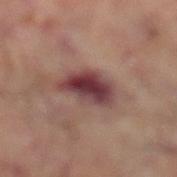- follow-up: catalogued during a skin exam; not biopsied
- image source: 15 mm crop, total-body photography
- location: the leg
- subject: male, aged 68 to 72
- illumination: cross-polarized